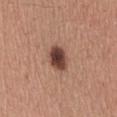Part of a total-body skin-imaging series; this lesion was reviewed on a skin check and was not flagged for biopsy. The recorded lesion diameter is about 3.5 mm. This image is a 15 mm lesion crop taken from a total-body photograph. The tile uses white-light illumination. Automated image analysis of the tile measured an average lesion color of about L≈43 a*≈20 b*≈25 (CIELAB) and a lesion–skin lightness drop of about 16. And it measured an automated nevus-likeness rating near 80 out of 100 and lesion-presence confidence of about 100/100. From the left forearm. A female patient, aged around 55.